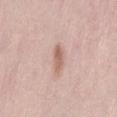About 3 mm across.
The tile uses white-light illumination.
The lesion is on the mid back.
A female patient, approximately 50 years of age.
A lesion tile, about 15 mm wide, cut from a 3D total-body photograph.